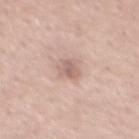Clinical impression:
The lesion was tiled from a total-body skin photograph and was not biopsied.
Background:
Imaged with white-light lighting. Located on the chest. Cropped from a whole-body photographic skin survey; the tile spans about 15 mm. The patient is a male roughly 55 years of age. The lesion-visualizer software estimated an area of roughly 4.5 mm², an eccentricity of roughly 0.6, and a symmetry-axis asymmetry near 0.25. And it measured a border-irregularity rating of about 2.5/10 and a peripheral color-asymmetry measure near 1. It also reported a nevus-likeness score of about 0/100.